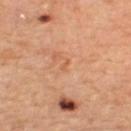Diagnosis: On biopsy, histopathology showed a lentigo.